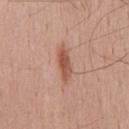Impression:
Imaged during a routine full-body skin examination; the lesion was not biopsied and no histopathology is available.
Clinical summary:
A male subject about 55 years old. A close-up tile cropped from a whole-body skin photograph, about 15 mm across. Captured under white-light illumination.